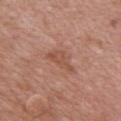No biopsy was performed on this lesion — it was imaged during a full skin examination and was not determined to be concerning.
The tile uses white-light illumination.
The patient is a male aged 63–67.
Approximately 4 mm at its widest.
On the chest.
A lesion tile, about 15 mm wide, cut from a 3D total-body photograph.
The lesion-visualizer software estimated an average lesion color of about L≈52 a*≈23 b*≈29 (CIELAB), a lesion–skin lightness drop of about 7, and a normalized lesion–skin contrast near 5.5. It also reported a color-variation rating of about 2/10 and peripheral color asymmetry of about 0.5. The analysis additionally found a classifier nevus-likeness of about 0/100 and lesion-presence confidence of about 100/100.